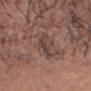Q: Was a biopsy performed?
A: imaged on a skin check; not biopsied
Q: Lesion location?
A: the head or neck
Q: What is the imaging modality?
A: total-body-photography crop, ~15 mm field of view
Q: Patient demographics?
A: male, in their mid-50s
Q: Lesion size?
A: about 4.5 mm
Q: How was the tile lit?
A: white-light illumination
Q: What did automated image analysis measure?
A: a footprint of about 6.5 mm², an eccentricity of roughly 0.85, and two-axis asymmetry of about 0.35; a color-variation rating of about 2.5/10 and radial color variation of about 0.5